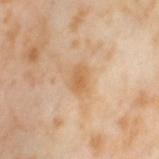Case summary:
• biopsy status — total-body-photography surveillance lesion; no biopsy
• subject — female, aged around 55
• illumination — cross-polarized
• body site — the leg
• lesion diameter — ~2.5 mm (longest diameter)
• image source — 15 mm crop, total-body photography